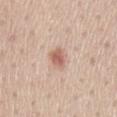{
  "biopsy_status": "not biopsied; imaged during a skin examination",
  "lighting": "white-light",
  "image": {
    "source": "total-body photography crop",
    "field_of_view_mm": 15
  },
  "patient": {
    "sex": "male",
    "age_approx": 60
  },
  "lesion_size": {
    "long_diameter_mm_approx": 2.5
  },
  "site": "mid back"
}